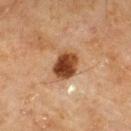Q: What did automated image analysis measure?
A: a footprint of about 8.5 mm², an outline eccentricity of about 0.55 (0 = round, 1 = elongated), and two-axis asymmetry of about 0.2; internal color variation of about 5 on a 0–10 scale and a peripheral color-asymmetry measure near 2
Q: What is the imaging modality?
A: total-body-photography crop, ~15 mm field of view
Q: Lesion location?
A: the upper back
Q: What are the patient's age and sex?
A: male, aged around 70
Q: How was the tile lit?
A: cross-polarized